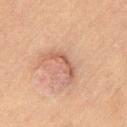biopsy status = catalogued during a skin exam; not biopsied | location = the leg | imaging modality = ~15 mm tile from a whole-body skin photo | subject = male, aged around 70.